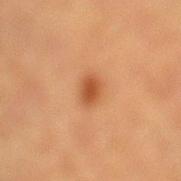Q: Lesion location?
A: the leg
Q: Lesion size?
A: about 2.5 mm
Q: Automated lesion metrics?
A: an area of roughly 4 mm² and a shape-asymmetry score of about 0.15 (0 = symmetric); border irregularity of about 1.5 on a 0–10 scale and peripheral color asymmetry of about 0.5; lesion-presence confidence of about 100/100
Q: What kind of image is this?
A: total-body-photography crop, ~15 mm field of view
Q: Illumination type?
A: cross-polarized
Q: Patient demographics?
A: male, about 85 years old or older